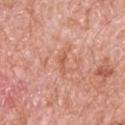Notes:
– workup · imaged on a skin check; not biopsied
– automated metrics · a mean CIELAB color near L≈58 a*≈27 b*≈34 and a lesion–skin lightness drop of about 7
– lesion diameter · ≈2.5 mm
– illumination · white-light
– subject · male, about 80 years old
– location · the upper back
– image source · ~15 mm tile from a whole-body skin photo